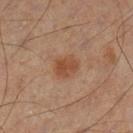Part of a total-body skin-imaging series; this lesion was reviewed on a skin check and was not flagged for biopsy.
A roughly 15 mm field-of-view crop from a total-body skin photograph.
The subject is a male roughly 65 years of age.
On the left lower leg.